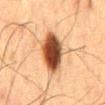The lesion was photographed on a routine skin check and not biopsied; there is no pathology result. The recorded lesion diameter is about 5.5 mm. On the mid back. The patient is a male aged 58–62. A close-up tile cropped from a whole-body skin photograph, about 15 mm across. Automated tile analysis of the lesion measured radial color variation of about 2. And it measured a classifier nevus-likeness of about 100/100 and a detector confidence of about 100 out of 100 that the crop contains a lesion. Imaged with cross-polarized lighting.